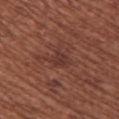{
  "biopsy_status": "not biopsied; imaged during a skin examination",
  "patient": {
    "sex": "female",
    "age_approx": 65
  },
  "image": {
    "source": "total-body photography crop",
    "field_of_view_mm": 15
  },
  "lesion_size": {
    "long_diameter_mm_approx": 3.0
  },
  "site": "upper back"
}